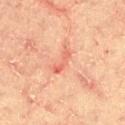follow-up=imaged on a skin check; not biopsied | image=total-body-photography crop, ~15 mm field of view | body site=the right thigh | patient=male, aged 63 to 67.